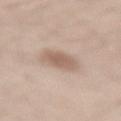<record>
<biopsy_status>not biopsied; imaged during a skin examination</biopsy_status>
<patient>
  <sex>male</sex>
  <age_approx>45</age_approx>
</patient>
<site>right thigh</site>
<image>
  <source>total-body photography crop</source>
  <field_of_view_mm>15</field_of_view_mm>
</image>
<lighting>white-light</lighting>
</record>